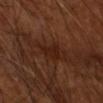Imaged during a routine full-body skin examination; the lesion was not biopsied and no histopathology is available. Captured under cross-polarized illumination. The lesion is located on the head or neck. A male subject, aged 58–62. This image is a 15 mm lesion crop taken from a total-body photograph.